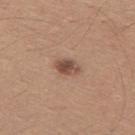follow-up — total-body-photography surveillance lesion; no biopsy
body site — the mid back
patient — male, approximately 30 years of age
image source — ~15 mm crop, total-body skin-cancer survey